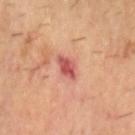This lesion was catalogued during total-body skin photography and was not selected for biopsy.
The lesion is on the head or neck.
A 15 mm crop from a total-body photograph taken for skin-cancer surveillance.
Captured under cross-polarized illumination.
The patient is a male approximately 55 years of age.